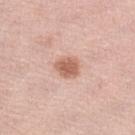biopsy status: no biopsy performed (imaged during a skin exam)
imaging modality: ~15 mm tile from a whole-body skin photo
location: the left lower leg
size: ~3 mm (longest diameter)
tile lighting: white-light illumination
patient: female, in their mid- to late 60s